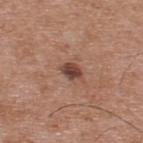Assessment:
Captured during whole-body skin photography for melanoma surveillance; the lesion was not biopsied.
Clinical summary:
A 15 mm crop from a total-body photograph taken for skin-cancer surveillance. The tile uses white-light illumination. Measured at roughly 2.5 mm in maximum diameter. The patient is a male aged approximately 55. From the upper back.